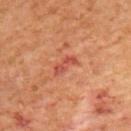Imaged during a routine full-body skin examination; the lesion was not biopsied and no histopathology is available.
A close-up tile cropped from a whole-body skin photograph, about 15 mm across.
The subject is a female in their 50s.
Approximately 3 mm at its widest.
Automated tile analysis of the lesion measured a lesion area of about 3.5 mm², an eccentricity of roughly 0.9, and a symmetry-axis asymmetry near 0.4. The analysis additionally found a within-lesion color-variation index near 0/10. It also reported a detector confidence of about 100 out of 100 that the crop contains a lesion.
The lesion is located on the left upper arm.
Imaged with cross-polarized lighting.